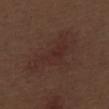The lesion was photographed on a routine skin check and not biopsied; there is no pathology result. Captured under white-light illumination. Cropped from a whole-body photographic skin survey; the tile spans about 15 mm. The lesion is located on the right thigh. A male subject, roughly 70 years of age. The lesion's longest dimension is about 8.5 mm. Automated image analysis of the tile measured a footprint of about 19 mm², an eccentricity of roughly 0.9, and a shape-asymmetry score of about 0.45 (0 = symmetric). And it measured a mean CIELAB color near L≈29 a*≈18 b*≈21, roughly 5 lightness units darker than nearby skin, and a lesion-to-skin contrast of about 5 (normalized; higher = more distinct). The analysis additionally found internal color variation of about 2.5 on a 0–10 scale and a peripheral color-asymmetry measure near 1.